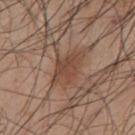The lesion was tiled from a total-body skin photograph and was not biopsied.